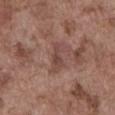| key | value |
|---|---|
| notes | catalogued during a skin exam; not biopsied |
| illumination | white-light |
| acquisition | 15 mm crop, total-body photography |
| automated metrics | a lesion color around L≈45 a*≈19 b*≈23 in CIELAB and a normalized border contrast of about 6.5; a border-irregularity rating of about 5/10 |
| body site | the abdomen |
| subject | male, roughly 75 years of age |
| size | about 4 mm |